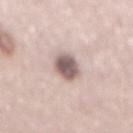  biopsy_status: not biopsied; imaged during a skin examination
  patient:
    sex: female
    age_approx: 50
  site: back
  lighting: white-light
  automated_metrics:
    eccentricity: 0.85
    shape_asymmetry: 0.2
    cielab_L: 59
    cielab_a: 16
    cielab_b: 19
    vs_skin_contrast_norm: 10.5
    border_irregularity_0_10: 2.0
    peripheral_color_asymmetry: 1.0
    nevus_likeness_0_100: 65
    lesion_detection_confidence_0_100: 90
  image:
    source: total-body photography crop
    field_of_view_mm: 15